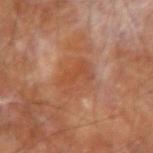The lesion was tiled from a total-body skin photograph and was not biopsied. Captured under cross-polarized illumination. About 4 mm across. Cropped from a total-body skin-imaging series; the visible field is about 15 mm. A male patient roughly 65 years of age. On the arm.